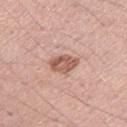| key | value |
|---|---|
| diameter | ~3.5 mm (longest diameter) |
| automated metrics | a lesion area of about 6 mm² and a shape-asymmetry score of about 0.2 (0 = symmetric); an average lesion color of about L≈57 a*≈23 b*≈28 (CIELAB) and a lesion–skin lightness drop of about 13 |
| image source | ~15 mm tile from a whole-body skin photo |
| subject | male, roughly 50 years of age |
| tile lighting | white-light illumination |
| body site | the right upper arm |